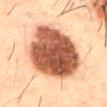– subject · male, aged 48 to 52
– lesion size · about 8.5 mm
– image source · ~15 mm crop, total-body skin-cancer survey
– anatomic site · the mid back
– lighting · cross-polarized illumination
– automated metrics · a shape eccentricity near 0.65 and two-axis asymmetry of about 0.15; a lesion color around L≈43 a*≈21 b*≈28 in CIELAB, a lesion–skin lightness drop of about 21, and a lesion-to-skin contrast of about 14.5 (normalized; higher = more distinct); border irregularity of about 1.5 on a 0–10 scale, a color-variation rating of about 6/10, and a peripheral color-asymmetry measure near 2; an automated nevus-likeness rating near 75 out of 100 and a detector confidence of about 100 out of 100 that the crop contains a lesion